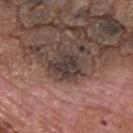Clinical impression:
Recorded during total-body skin imaging; not selected for excision or biopsy.
Background:
The tile uses white-light illumination. The total-body-photography lesion software estimated roughly 11 lightness units darker than nearby skin and a normalized lesion–skin contrast near 10. The analysis additionally found border irregularity of about 3.5 on a 0–10 scale and radial color variation of about 1. Cropped from a whole-body photographic skin survey; the tile spans about 15 mm. A male patient in their mid- to late 70s. On the upper back. The lesion's longest dimension is about 3.5 mm.